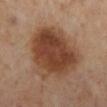Recorded during total-body skin imaging; not selected for excision or biopsy. The lesion is on the left lower leg. A female subject about 55 years old. Approximately 7.5 mm at its widest. A 15 mm crop from a total-body photograph taken for skin-cancer surveillance. Captured under cross-polarized illumination. The lesion-visualizer software estimated a lesion color around L≈43 a*≈21 b*≈31 in CIELAB, about 13 CIELAB-L* units darker than the surrounding skin, and a lesion-to-skin contrast of about 10 (normalized; higher = more distinct). The software also gave a nevus-likeness score of about 100/100 and lesion-presence confidence of about 100/100.